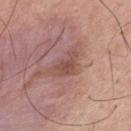Captured during whole-body skin photography for melanoma surveillance; the lesion was not biopsied.
The lesion's longest dimension is about 4 mm.
The lesion is located on the upper back.
A male patient aged 53 to 57.
Cropped from a whole-body photographic skin survey; the tile spans about 15 mm.